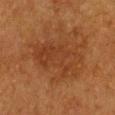Captured during whole-body skin photography for melanoma surveillance; the lesion was not biopsied.
The subject is a female in their 40s.
About 7 mm across.
An algorithmic analysis of the crop reported roughly 5 lightness units darker than nearby skin and a lesion-to-skin contrast of about 5.5 (normalized; higher = more distinct). The software also gave a border-irregularity index near 5/10.
Located on the chest.
A 15 mm close-up extracted from a 3D total-body photography capture.